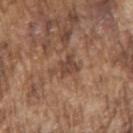Imaged during a routine full-body skin examination; the lesion was not biopsied and no histopathology is available.
Located on the right upper arm.
A male patient, aged around 75.
A 15 mm close-up extracted from a 3D total-body photography capture.